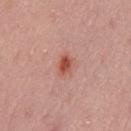<tbp_lesion>
<biopsy_status>not biopsied; imaged during a skin examination</biopsy_status>
<automated_metrics>
  <area_mm2_approx>4.5</area_mm2_approx>
  <eccentricity>0.65</eccentricity>
  <shape_asymmetry>0.25</shape_asymmetry>
  <border_irregularity_0_10>2.0</border_irregularity_0_10>
  <color_variation_0_10>5.0</color_variation_0_10>
  <peripheral_color_asymmetry>1.5</peripheral_color_asymmetry>
  <nevus_likeness_0_100>90</nevus_likeness_0_100>
  <lesion_detection_confidence_0_100>100</lesion_detection_confidence_0_100>
</automated_metrics>
<lighting>white-light</lighting>
<patient>
  <sex>male</sex>
  <age_approx>40</age_approx>
</patient>
<lesion_size>
  <long_diameter_mm_approx>2.5</long_diameter_mm_approx>
</lesion_size>
<image>
  <source>total-body photography crop</source>
  <field_of_view_mm>15</field_of_view_mm>
</image>
<site>left thigh</site>
</tbp_lesion>